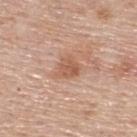Assessment: The lesion was photographed on a routine skin check and not biopsied; there is no pathology result. Image and clinical context: On the upper back. A lesion tile, about 15 mm wide, cut from a 3D total-body photograph. About 3 mm across. A female subject about 65 years old.